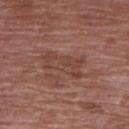Assessment:
Part of a total-body skin-imaging series; this lesion was reviewed on a skin check and was not flagged for biopsy.
Context:
A female patient about 70 years old. A close-up tile cropped from a whole-body skin photograph, about 15 mm across. From the right upper arm. Longest diameter approximately 5 mm. The lesion-visualizer software estimated a lesion area of about 9.5 mm², an outline eccentricity of about 0.9 (0 = round, 1 = elongated), and two-axis asymmetry of about 0.3. The software also gave a border-irregularity index near 4.5/10, a within-lesion color-variation index near 3/10, and peripheral color asymmetry of about 1. Captured under white-light illumination.